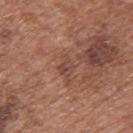Clinical summary:
The recorded lesion diameter is about 2.5 mm. Automated image analysis of the tile measured a footprint of about 3.5 mm² and a shape-asymmetry score of about 0.35 (0 = symmetric). It also reported a lesion color around L≈45 a*≈21 b*≈25 in CIELAB, a lesion–skin lightness drop of about 7, and a normalized border contrast of about 6. And it measured lesion-presence confidence of about 95/100. The tile uses white-light illumination. A roughly 15 mm field-of-view crop from a total-body skin photograph. The lesion is located on the upper back. A male patient, roughly 75 years of age.